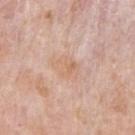A close-up tile cropped from a whole-body skin photograph, about 15 mm across. The subject is a male roughly 75 years of age. From the arm.